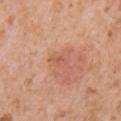{
  "biopsy_status": "not biopsied; imaged during a skin examination",
  "patient": {
    "sex": "female",
    "age_approx": 40
  },
  "image": {
    "source": "total-body photography crop",
    "field_of_view_mm": 15
  },
  "site": "right upper arm"
}